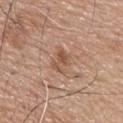Assessment:
This lesion was catalogued during total-body skin photography and was not selected for biopsy.
Background:
A male subject aged 73 to 77. The lesion is located on the upper back. A lesion tile, about 15 mm wide, cut from a 3D total-body photograph.